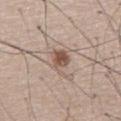Q: What are the patient's age and sex?
A: male, aged approximately 55
Q: Illumination type?
A: white-light illumination
Q: What did automated image analysis measure?
A: a classifier nevus-likeness of about 90/100 and a detector confidence of about 100 out of 100 that the crop contains a lesion
Q: How was this image acquired?
A: 15 mm crop, total-body photography
Q: Lesion location?
A: the front of the torso
Q: What is the lesion's diameter?
A: ≈4 mm